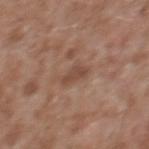The lesion is located on the upper back. A male subject, aged 43–47. Approximately 3 mm at its widest. Imaged with white-light lighting. A close-up tile cropped from a whole-body skin photograph, about 15 mm across.